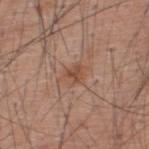Imaged during a routine full-body skin examination; the lesion was not biopsied and no histopathology is available. Longest diameter approximately 3.5 mm. A roughly 15 mm field-of-view crop from a total-body skin photograph. The lesion is located on the upper back. The patient is a male aged around 60.